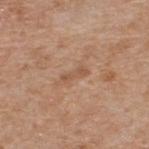Impression:
The lesion was photographed on a routine skin check and not biopsied; there is no pathology result.
Acquisition and patient details:
A male patient about 60 years old. Automated tile analysis of the lesion measured a border-irregularity rating of about 3.5/10, a color-variation rating of about 0/10, and a peripheral color-asymmetry measure near 0. The analysis additionally found an automated nevus-likeness rating near 0 out of 100. A 15 mm close-up tile from a total-body photography series done for melanoma screening. The lesion is located on the upper back. Imaged with white-light lighting.